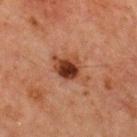body site: the chest; acquisition: total-body-photography crop, ~15 mm field of view; patient: male, approximately 65 years of age.